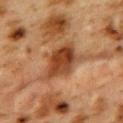Part of a total-body skin-imaging series; this lesion was reviewed on a skin check and was not flagged for biopsy. On the upper back. Imaged with cross-polarized lighting. A female subject, in their 40s. A region of skin cropped from a whole-body photographic capture, roughly 15 mm wide. The total-body-photography lesion software estimated a border-irregularity rating of about 4/10 and radial color variation of about 1.5. The analysis additionally found lesion-presence confidence of about 100/100.